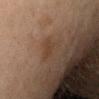Captured during whole-body skin photography for melanoma surveillance; the lesion was not biopsied.
A close-up tile cropped from a whole-body skin photograph, about 15 mm across.
Located on the right upper arm.
A female patient, aged 58 to 62.
The total-body-photography lesion software estimated a shape eccentricity near 0.9 and two-axis asymmetry of about 0.25. The software also gave a border-irregularity index near 3/10 and radial color variation of about 0.5.
The tile uses cross-polarized illumination.
Approximately 3 mm at its widest.